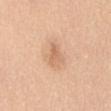biopsy_status: not biopsied; imaged during a skin examination
site: mid back
image:
  source: total-body photography crop
  field_of_view_mm: 15
lesion_size:
  long_diameter_mm_approx: 3.5
lighting: white-light
patient:
  sex: female
  age_approx: 55